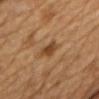Clinical impression:
The lesion was photographed on a routine skin check and not biopsied; there is no pathology result.
Context:
Approximately 3.5 mm at its widest. A male patient, aged approximately 85 or older. Imaged with cross-polarized lighting. Located on the chest. A 15 mm crop from a total-body photograph taken for skin-cancer surveillance.